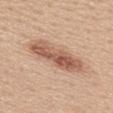Q: Was a biopsy performed?
A: no biopsy performed (imaged during a skin exam)
Q: Patient demographics?
A: female, roughly 35 years of age
Q: What kind of image is this?
A: ~15 mm crop, total-body skin-cancer survey
Q: Automated lesion metrics?
A: a lesion area of about 15 mm², an outline eccentricity of about 0.95 (0 = round, 1 = elongated), and a shape-asymmetry score of about 0.2 (0 = symmetric); a border-irregularity index near 3.5/10; a nevus-likeness score of about 35/100 and a detector confidence of about 100 out of 100 that the crop contains a lesion
Q: Lesion location?
A: the upper back